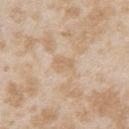The lesion was photographed on a routine skin check and not biopsied; there is no pathology result. An algorithmic analysis of the crop reported a lesion color around L≈66 a*≈14 b*≈33 in CIELAB, about 6 CIELAB-L* units darker than the surrounding skin, and a normalized border contrast of about 5. It also reported a border-irregularity rating of about 2/10, internal color variation of about 1.5 on a 0–10 scale, and peripheral color asymmetry of about 0.5. On the right upper arm. The tile uses white-light illumination. A close-up tile cropped from a whole-body skin photograph, about 15 mm across. The lesion's longest dimension is about 2.5 mm. The subject is a female about 25 years old.